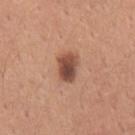biopsy status: catalogued during a skin exam; not biopsied
TBP lesion metrics: an area of roughly 6.5 mm² and a symmetry-axis asymmetry near 0.3; a border-irregularity rating of about 2.5/10, a within-lesion color-variation index near 6/10, and a peripheral color-asymmetry measure near 2
lesion size: ~3.5 mm (longest diameter)
subject: male, roughly 30 years of age
body site: the right upper arm
image source: total-body-photography crop, ~15 mm field of view
illumination: white-light